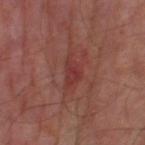Case summary:
- notes — catalogued during a skin exam; not biopsied
- acquisition — 15 mm crop, total-body photography
- lesion size — about 4 mm
- subject — male, in their mid- to late 60s
- site — the left thigh
- tile lighting — cross-polarized illumination